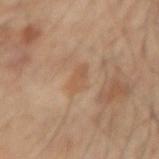The lesion was photographed on a routine skin check and not biopsied; there is no pathology result.
The recorded lesion diameter is about 3 mm.
A 15 mm close-up tile from a total-body photography series done for melanoma screening.
The subject is a male aged approximately 55.
From the right forearm.